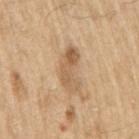Clinical impression: Captured during whole-body skin photography for melanoma surveillance; the lesion was not biopsied. Background: A 15 mm crop from a total-body photograph taken for skin-cancer surveillance. On the right upper arm. Approximately 5 mm at its widest. The patient is a male in their 70s.